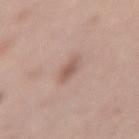The lesion was tiled from a total-body skin photograph and was not biopsied. On the left thigh. Automated image analysis of the tile measured a peripheral color-asymmetry measure near 0. The lesion's longest dimension is about 3.5 mm. This is a white-light tile. A female subject aged 38 to 42. A close-up tile cropped from a whole-body skin photograph, about 15 mm across.